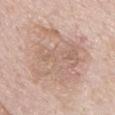Impression: The lesion was photographed on a routine skin check and not biopsied; there is no pathology result. Background: Located on the chest. The lesion's longest dimension is about 8.5 mm. This image is a 15 mm lesion crop taken from a total-body photograph. This is a white-light tile. A male subject aged approximately 75.